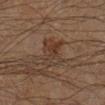Part of a total-body skin-imaging series; this lesion was reviewed on a skin check and was not flagged for biopsy. Imaged with cross-polarized lighting. On the right lower leg. The subject is a male in their mid- to late 60s. The lesion's longest dimension is about 4 mm. A roughly 15 mm field-of-view crop from a total-body skin photograph.